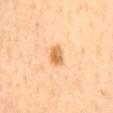Recorded during total-body skin imaging; not selected for excision or biopsy.
About 2.5 mm across.
On the mid back.
Automated image analysis of the tile measured a border-irregularity index near 1.5/10, a color-variation rating of about 4/10, and a peripheral color-asymmetry measure near 1.5. And it measured a classifier nevus-likeness of about 100/100.
Cropped from a total-body skin-imaging series; the visible field is about 15 mm.
A subject aged approximately 65.